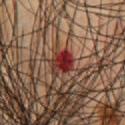illumination — cross-polarized; subject — male, aged around 45; body site — the chest; image source — 15 mm crop, total-body photography; diameter — ~3 mm (longest diameter).